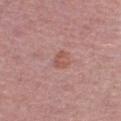| feature | finding |
|---|---|
| notes | catalogued during a skin exam; not biopsied |
| location | the right lower leg |
| illumination | white-light illumination |
| image | total-body-photography crop, ~15 mm field of view |
| lesion diameter | ≈2 mm |
| subject | female, aged approximately 50 |